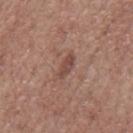Impression:
The lesion was tiled from a total-body skin photograph and was not biopsied.
Image and clinical context:
A male subject about 70 years old. A 15 mm crop from a total-body photograph taken for skin-cancer surveillance. The lesion is on the mid back. This is a white-light tile.